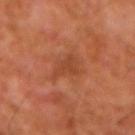Findings:
- biopsy status: no biopsy performed (imaged during a skin exam)
- body site: the left forearm
- image source: ~15 mm tile from a whole-body skin photo
- lesion diameter: ~4.5 mm (longest diameter)
- patient: male, aged around 60
- illumination: cross-polarized